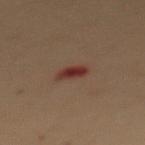Clinical impression: No biopsy was performed on this lesion — it was imaged during a full skin examination and was not determined to be concerning. Background: A 15 mm close-up tile from a total-body photography series done for melanoma screening. The recorded lesion diameter is about 2.5 mm. Imaged with cross-polarized lighting. From the upper back. A female subject, aged around 50.